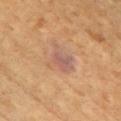  biopsy_status: not biopsied; imaged during a skin examination
  lighting: cross-polarized
  site: chest
  patient:
    sex: male
    age_approx: 55
  image:
    source: total-body photography crop
    field_of_view_mm: 15